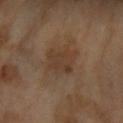The lesion was tiled from a total-body skin photograph and was not biopsied. The recorded lesion diameter is about 3.5 mm. A female patient, aged around 70. A roughly 15 mm field-of-view crop from a total-body skin photograph. The lesion is on the right forearm.